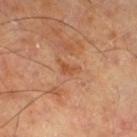<tbp_lesion>
  <lighting>cross-polarized</lighting>
  <image>
    <source>total-body photography crop</source>
    <field_of_view_mm>15</field_of_view_mm>
  </image>
  <site>right thigh</site>
  <lesion_size>
    <long_diameter_mm_approx>3.0</long_diameter_mm_approx>
  </lesion_size>
  <patient>
    <age_approx>65</age_approx>
  </patient>
</tbp_lesion>